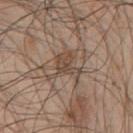Q: Was a biopsy performed?
A: no biopsy performed (imaged during a skin exam)
Q: How was the tile lit?
A: white-light illumination
Q: What is the lesion's diameter?
A: about 4 mm
Q: How was this image acquired?
A: 15 mm crop, total-body photography
Q: Automated lesion metrics?
A: an area of roughly 9 mm², an eccentricity of roughly 0.35, and a symmetry-axis asymmetry near 0.55; border irregularity of about 7.5 on a 0–10 scale and a color-variation rating of about 4/10
Q: Where on the body is the lesion?
A: the chest
Q: What are the patient's age and sex?
A: male, aged approximately 45